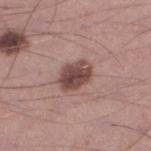This lesion was catalogued during total-body skin photography and was not selected for biopsy. A male patient, about 35 years old. About 4 mm across. The lesion-visualizer software estimated a shape eccentricity near 0.7 and a shape-asymmetry score of about 0.2 (0 = symmetric). And it measured a lesion color around L≈46 a*≈20 b*≈21 in CIELAB and about 14 CIELAB-L* units darker than the surrounding skin. A 15 mm close-up extracted from a 3D total-body photography capture. The lesion is located on the left lower leg.